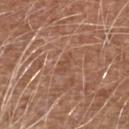The lesion was tiled from a total-body skin photograph and was not biopsied.